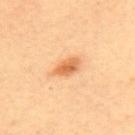<case>
<patient>
  <sex>female</sex>
  <age_approx>35</age_approx>
</patient>
<site>back</site>
<automated_metrics>
  <area_mm2_approx>4.5</area_mm2_approx>
  <eccentricity>0.85</eccentricity>
  <shape_asymmetry>0.2</shape_asymmetry>
  <vs_skin_darker_L>13.0</vs_skin_darker_L>
  <vs_skin_contrast_norm>8.0</vs_skin_contrast_norm>
  <nevus_likeness_0_100>95</nevus_likeness_0_100>
  <lesion_detection_confidence_0_100>100</lesion_detection_confidence_0_100>
</automated_metrics>
<image>
  <source>total-body photography crop</source>
  <field_of_view_mm>15</field_of_view_mm>
</image>
<lighting>cross-polarized</lighting>
</case>